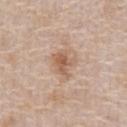notes=total-body-photography surveillance lesion; no biopsy
patient=female, aged approximately 60
anatomic site=the front of the torso
acquisition=~15 mm tile from a whole-body skin photo